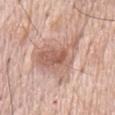Notes:
* notes · imaged on a skin check; not biopsied
* anatomic site · the chest
* subject · male, in their 70s
* illumination · white-light
* diameter · ~7 mm (longest diameter)
* imaging modality · total-body-photography crop, ~15 mm field of view
* automated lesion analysis · a border-irregularity rating of about 6/10, a color-variation rating of about 5.5/10, and peripheral color asymmetry of about 2